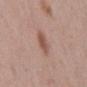Recorded during total-body skin imaging; not selected for excision or biopsy. The subject is a male about 70 years old. The lesion is on the mid back. A 15 mm close-up tile from a total-body photography series done for melanoma screening.